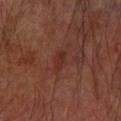Acquisition and patient details: The lesion is located on the left forearm. Automated tile analysis of the lesion measured a lesion–skin lightness drop of about 5 and a normalized border contrast of about 6. It also reported border irregularity of about 2.5 on a 0–10 scale, internal color variation of about 0.5 on a 0–10 scale, and a peripheral color-asymmetry measure near 0. The software also gave a classifier nevus-likeness of about 0/100 and a lesion-detection confidence of about 100/100. A lesion tile, about 15 mm wide, cut from a 3D total-body photograph. This is a cross-polarized tile. The lesion's longest dimension is about 2.5 mm. The subject is a male roughly 65 years of age.